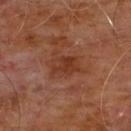notes: catalogued during a skin exam; not biopsied | anatomic site: the chest | automated metrics: a border-irregularity index near 2.5/10, a color-variation rating of about 3.5/10, and radial color variation of about 1 | patient: male, approximately 60 years of age | image: ~15 mm crop, total-body skin-cancer survey | lesion size: ≈3.5 mm.